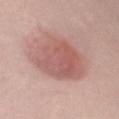Assessment: Imaged during a routine full-body skin examination; the lesion was not biopsied and no histopathology is available. Background: The lesion's longest dimension is about 9.5 mm. A female patient, aged 38–42. Automated tile analysis of the lesion measured an area of roughly 28 mm², an outline eccentricity of about 0.9 (0 = round, 1 = elongated), and a symmetry-axis asymmetry near 0.2. The software also gave border irregularity of about 3 on a 0–10 scale, a color-variation rating of about 4.5/10, and radial color variation of about 1.5. Imaged with white-light lighting. A 15 mm close-up tile from a total-body photography series done for melanoma screening. Located on the mid back.